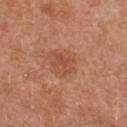<tbp_lesion>
  <biopsy_status>not biopsied; imaged during a skin examination</biopsy_status>
</tbp_lesion>